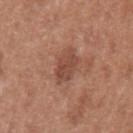Clinical impression:
The lesion was tiled from a total-body skin photograph and was not biopsied.
Acquisition and patient details:
A female subject, aged around 50. The lesion is on the arm. A close-up tile cropped from a whole-body skin photograph, about 15 mm across. Automated tile analysis of the lesion measured an average lesion color of about L≈47 a*≈23 b*≈28 (CIELAB), a lesion–skin lightness drop of about 9, and a lesion-to-skin contrast of about 6.5 (normalized; higher = more distinct). The software also gave peripheral color asymmetry of about 1. It also reported lesion-presence confidence of about 100/100. Approximately 3.5 mm at its widest. This is a white-light tile.